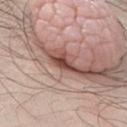A 15 mm close-up extracted from a 3D total-body photography capture.
A male patient in their mid-40s.
On the abdomen.